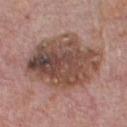biopsy status: total-body-photography surveillance lesion; no biopsy
patient: male, in their mid- to late 70s
diameter: ~9.5 mm (longest diameter)
acquisition: ~15 mm crop, total-body skin-cancer survey
location: the chest